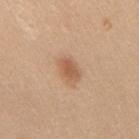Q: Was this lesion biopsied?
A: no biopsy performed (imaged during a skin exam)
Q: What is the anatomic site?
A: the back
Q: How was this image acquired?
A: ~15 mm crop, total-body skin-cancer survey
Q: Who is the patient?
A: female, aged around 40